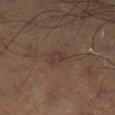Q: Was this lesion biopsied?
A: total-body-photography surveillance lesion; no biopsy
Q: What lighting was used for the tile?
A: cross-polarized
Q: Where on the body is the lesion?
A: the left lower leg
Q: What did automated image analysis measure?
A: an area of roughly 4 mm², an eccentricity of roughly 0.75, and two-axis asymmetry of about 0.3; an average lesion color of about L≈35 a*≈16 b*≈21 (CIELAB) and a lesion-to-skin contrast of about 5 (normalized; higher = more distinct); a border-irregularity index near 2.5/10 and peripheral color asymmetry of about 0.5
Q: What is the imaging modality?
A: ~15 mm crop, total-body skin-cancer survey
Q: Patient demographics?
A: male, aged 43 to 47